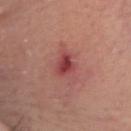Impression:
Recorded during total-body skin imaging; not selected for excision or biopsy.
Acquisition and patient details:
Approximately 2.5 mm at its widest. A male subject aged 63–67. From the head or neck. A 15 mm close-up extracted from a 3D total-body photography capture.